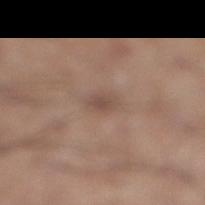Q: Was a biopsy performed?
A: imaged on a skin check; not biopsied
Q: Where on the body is the lesion?
A: the leg
Q: What are the patient's age and sex?
A: male, aged 58 to 62
Q: What is the imaging modality?
A: ~15 mm tile from a whole-body skin photo